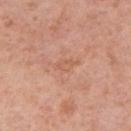Recorded during total-body skin imaging; not selected for excision or biopsy.
Cropped from a whole-body photographic skin survey; the tile spans about 15 mm.
On the right upper arm.
The patient is a female aged around 60.
This is a white-light tile.
Approximately 3 mm at its widest.